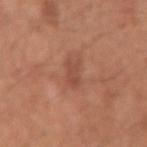Clinical impression: This lesion was catalogued during total-body skin photography and was not selected for biopsy. Acquisition and patient details: Located on the right upper arm. Cropped from a whole-body photographic skin survey; the tile spans about 15 mm. Approximately 3 mm at its widest. Automated image analysis of the tile measured a footprint of about 4.5 mm² and two-axis asymmetry of about 0.3. And it measured a border-irregularity rating of about 3/10, internal color variation of about 2 on a 0–10 scale, and a peripheral color-asymmetry measure near 0.5. And it measured a nevus-likeness score of about 0/100 and a detector confidence of about 100 out of 100 that the crop contains a lesion. The subject is a male roughly 55 years of age. Captured under white-light illumination.